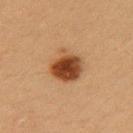Recorded during total-body skin imaging; not selected for excision or biopsy. Imaged with cross-polarized lighting. A female patient, approximately 40 years of age. The lesion-visualizer software estimated a shape eccentricity near 0.3 and two-axis asymmetry of about 0.15. It also reported a border-irregularity rating of about 1.5/10 and radial color variation of about 1.5. The analysis additionally found a classifier nevus-likeness of about 100/100 and lesion-presence confidence of about 100/100. A 15 mm close-up extracted from a 3D total-body photography capture. From the left upper arm. Longest diameter approximately 4 mm.